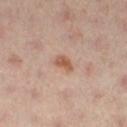| field | value |
|---|---|
| notes | total-body-photography surveillance lesion; no biopsy |
| illumination | cross-polarized illumination |
| automated lesion analysis | an average lesion color of about L≈57 a*≈22 b*≈31 (CIELAB); a border-irregularity index near 3.5/10 and internal color variation of about 3 on a 0–10 scale; lesion-presence confidence of about 100/100 |
| patient | female, in their 40s |
| imaging modality | 15 mm crop, total-body photography |
| size | about 2.5 mm |
| body site | the right leg |